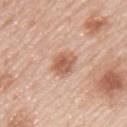The lesion was photographed on a routine skin check and not biopsied; there is no pathology result.
Approximately 3.5 mm at its widest.
A 15 mm crop from a total-body photograph taken for skin-cancer surveillance.
A male patient approximately 45 years of age.
From the upper back.
Automated image analysis of the tile measured a mean CIELAB color near L≈60 a*≈23 b*≈31, a lesion–skin lightness drop of about 12, and a lesion-to-skin contrast of about 8 (normalized; higher = more distinct). The analysis additionally found a classifier nevus-likeness of about 90/100.
Imaged with white-light lighting.